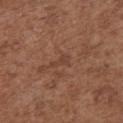{"biopsy_status": "not biopsied; imaged during a skin examination", "lesion_size": {"long_diameter_mm_approx": 2.5}, "patient": {"sex": "female", "age_approx": 75}, "image": {"source": "total-body photography crop", "field_of_view_mm": 15}, "lighting": "white-light", "site": "chest"}